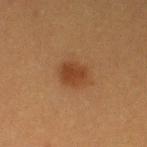Q: Was this lesion biopsied?
A: no biopsy performed (imaged during a skin exam)